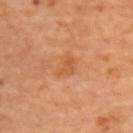Clinical summary:
On the upper back. A female subject, aged 68 to 72. A 15 mm crop from a total-body photograph taken for skin-cancer surveillance.